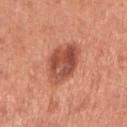Part of a total-body skin-imaging series; this lesion was reviewed on a skin check and was not flagged for biopsy.
From the right upper arm.
A region of skin cropped from a whole-body photographic capture, roughly 15 mm wide.
A female subject approximately 50 years of age.